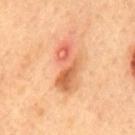notes = total-body-photography surveillance lesion; no biopsy
subject = male, aged 48 to 52
TBP lesion metrics = an area of roughly 13 mm²; a border-irregularity index near 4/10, a within-lesion color-variation index near 10/10, and radial color variation of about 3.5
image = ~15 mm crop, total-body skin-cancer survey
tile lighting = cross-polarized
size = about 6 mm
body site = the back